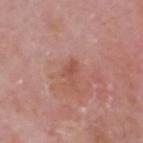The lesion was tiled from a total-body skin photograph and was not biopsied. The subject is a male aged 58–62. Imaged with white-light lighting. A region of skin cropped from a whole-body photographic capture, roughly 15 mm wide. The lesion-visualizer software estimated an average lesion color of about L≈52 a*≈25 b*≈29 (CIELAB), a lesion–skin lightness drop of about 7, and a normalized lesion–skin contrast near 6. It also reported a border-irregularity rating of about 5.5/10. On the head or neck. The recorded lesion diameter is about 2.5 mm.